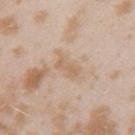<case>
<biopsy_status>not biopsied; imaged during a skin examination</biopsy_status>
<patient>
  <sex>female</sex>
  <age_approx>25</age_approx>
</patient>
<lesion_size>
  <long_diameter_mm_approx>3.0</long_diameter_mm_approx>
</lesion_size>
<image>
  <source>total-body photography crop</source>
  <field_of_view_mm>15</field_of_view_mm>
</image>
<site>arm</site>
<lighting>white-light</lighting>
</case>